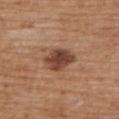Impression: The lesion was tiled from a total-body skin photograph and was not biopsied. Clinical summary: A roughly 15 mm field-of-view crop from a total-body skin photograph. The recorded lesion diameter is about 4 mm. A male subject, aged around 70. From the upper back. Captured under white-light illumination.